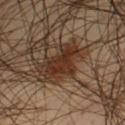biopsy_status: not biopsied; imaged during a skin examination
site: left thigh
image:
  source: total-body photography crop
  field_of_view_mm: 15
patient:
  sex: male
  age_approx: 45